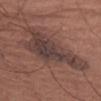Assessment:
The lesion was tiled from a total-body skin photograph and was not biopsied.
Acquisition and patient details:
From the abdomen. A female patient aged approximately 65. Captured under white-light illumination. A 15 mm close-up tile from a total-body photography series done for melanoma screening.